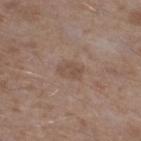Imaged during a routine full-body skin examination; the lesion was not biopsied and no histopathology is available.
The lesion's longest dimension is about 2.5 mm.
The subject is a male aged around 45.
From the left lower leg.
A 15 mm close-up extracted from a 3D total-body photography capture.
The tile uses white-light illumination.
Automated tile analysis of the lesion measured an area of roughly 4 mm² and an outline eccentricity of about 0.7 (0 = round, 1 = elongated).